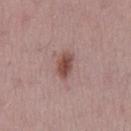Findings:
* notes — no biopsy performed (imaged during a skin exam)
* subject — male, approximately 70 years of age
* lesion size — ~3.5 mm (longest diameter)
* site — the mid back
* acquisition — ~15 mm crop, total-body skin-cancer survey